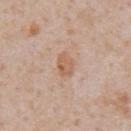workup: imaged on a skin check; not biopsied | patient: male, in their mid-60s | lesion size: ~3 mm (longest diameter) | image source: 15 mm crop, total-body photography | body site: the abdomen | lighting: white-light | image-analysis metrics: an area of roughly 5.5 mm², an outline eccentricity of about 0.7 (0 = round, 1 = elongated), and a shape-asymmetry score of about 0.15 (0 = symmetric); a lesion–skin lightness drop of about 8 and a lesion-to-skin contrast of about 6.5 (normalized; higher = more distinct); an automated nevus-likeness rating near 10 out of 100 and a lesion-detection confidence of about 100/100.